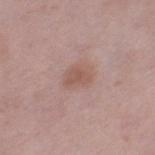biopsy status: no biopsy performed (imaged during a skin exam); subject: female, in their 40s; site: the left thigh; imaging modality: total-body-photography crop, ~15 mm field of view.